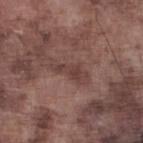Clinical impression: Captured during whole-body skin photography for melanoma surveillance; the lesion was not biopsied. Clinical summary: Approximately 4 mm at its widest. The lesion-visualizer software estimated an average lesion color of about L≈40 a*≈19 b*≈21 (CIELAB) and roughly 8 lightness units darker than nearby skin. A male subject, aged 73–77. Cropped from a total-body skin-imaging series; the visible field is about 15 mm. From the left lower leg.